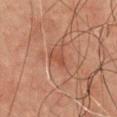Assessment: The lesion was photographed on a routine skin check and not biopsied; there is no pathology result.